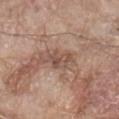biopsy_status: not biopsied; imaged during a skin examination
lesion_size:
  long_diameter_mm_approx: 3.5
lighting: white-light
automated_metrics:
  border_irregularity_0_10: 4.5
  color_variation_0_10: 4.0
  peripheral_color_asymmetry: 1.5
  nevus_likeness_0_100: 0
  lesion_detection_confidence_0_100: 100
patient:
  sex: male
  age_approx: 80
site: abdomen
image:
  source: total-body photography crop
  field_of_view_mm: 15